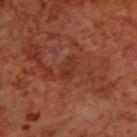Impression:
This lesion was catalogued during total-body skin photography and was not selected for biopsy.
Context:
Cropped from a whole-body photographic skin survey; the tile spans about 15 mm. The lesion's longest dimension is about 3 mm. The subject is a male about 70 years old. The lesion is located on the upper back. The tile uses cross-polarized illumination.